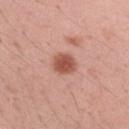diameter: ~2.5 mm (longest diameter) | patient: female, in their mid-30s | image: total-body-photography crop, ~15 mm field of view | anatomic site: the right upper arm | tile lighting: white-light | image-analysis metrics: a footprint of about 6 mm², a shape eccentricity near 0.45, and a shape-asymmetry score of about 0.2 (0 = symmetric); a border-irregularity index near 1.5/10, internal color variation of about 2.5 on a 0–10 scale, and radial color variation of about 0.5; a classifier nevus-likeness of about 95/100 and lesion-presence confidence of about 100/100.